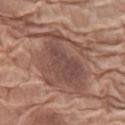– biopsy status — catalogued during a skin exam; not biopsied
– imaging modality — total-body-photography crop, ~15 mm field of view
– subject — female, in their mid- to late 70s
– body site — the left thigh